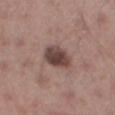follow-up: total-body-photography surveillance lesion; no biopsy
patient: male, in their mid-50s
automated lesion analysis: an area of roughly 8.5 mm², an eccentricity of roughly 0.7, and two-axis asymmetry of about 0.15; a nevus-likeness score of about 55/100
image source: 15 mm crop, total-body photography
tile lighting: white-light
site: the leg